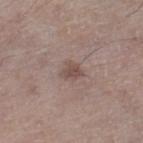follow-up=no biopsy performed (imaged during a skin exam) | subject=male, approximately 65 years of age | illumination=white-light | automated metrics=a footprint of about 4.5 mm² and a shape eccentricity near 0.45; a classifier nevus-likeness of about 35/100 and a lesion-detection confidence of about 100/100 | diameter=~2.5 mm (longest diameter) | imaging modality=total-body-photography crop, ~15 mm field of view | site=the right thigh.